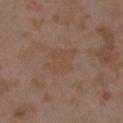Impression: Captured during whole-body skin photography for melanoma surveillance; the lesion was not biopsied. Background: Longest diameter approximately 4 mm. The tile uses white-light illumination. The patient is a female aged approximately 35. A 15 mm close-up extracted from a 3D total-body photography capture. The lesion is on the left upper arm.